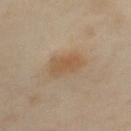• notes · total-body-photography surveillance lesion; no biopsy
• acquisition · ~15 mm crop, total-body skin-cancer survey
• lesion diameter · ~4.5 mm (longest diameter)
• location · the left upper arm
• subject · female, in their mid- to late 30s